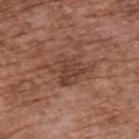Assessment:
Recorded during total-body skin imaging; not selected for excision or biopsy.
Background:
A region of skin cropped from a whole-body photographic capture, roughly 15 mm wide. The lesion is on the upper back. About 5.5 mm across. The patient is a male about 75 years old. The tile uses white-light illumination.